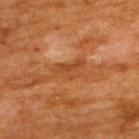{"biopsy_status": "not biopsied; imaged during a skin examination", "lesion_size": {"long_diameter_mm_approx": 4.0}, "image": {"source": "total-body photography crop", "field_of_view_mm": 15}, "site": "back", "patient": {"sex": "male", "age_approx": 60}}